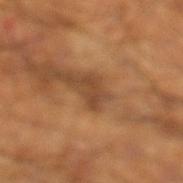Part of a total-body skin-imaging series; this lesion was reviewed on a skin check and was not flagged for biopsy. The lesion is on the left lower leg. This is a cross-polarized tile. A 15 mm crop from a total-body photograph taken for skin-cancer surveillance. Measured at roughly 2.5 mm in maximum diameter. The patient is a male aged around 60.